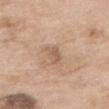biopsy_status: not biopsied; imaged during a skin examination
image:
  source: total-body photography crop
  field_of_view_mm: 15
lesion_size:
  long_diameter_mm_approx: 2.5
automated_metrics:
  cielab_L: 58
  cielab_a: 17
  cielab_b: 30
  vs_skin_darker_L: 8.0
patient:
  sex: female
  age_approx: 75
lighting: white-light
site: back